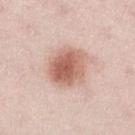{"biopsy_status": "not biopsied; imaged during a skin examination", "lesion_size": {"long_diameter_mm_approx": 5.0}, "site": "right lower leg", "image": {"source": "total-body photography crop", "field_of_view_mm": 15}, "patient": {"sex": "female", "age_approx": 30}}